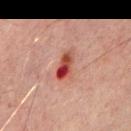Assessment:
No biopsy was performed on this lesion — it was imaged during a full skin examination and was not determined to be concerning.
Background:
Captured under cross-polarized illumination. An algorithmic analysis of the crop reported internal color variation of about 10 on a 0–10 scale and peripheral color asymmetry of about 6.5. Cropped from a whole-body photographic skin survey; the tile spans about 15 mm. The lesion is located on the mid back. About 4 mm across. A male patient, about 70 years old.